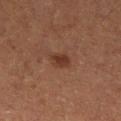diameter=≈2.5 mm | acquisition=15 mm crop, total-body photography | lighting=cross-polarized illumination | subject=female, approximately 50 years of age | anatomic site=the left lower leg | image-analysis metrics=a lesion color around L≈28 a*≈18 b*≈23 in CIELAB and about 7 CIELAB-L* units darker than the surrounding skin; a nevus-likeness score of about 85/100 and lesion-presence confidence of about 100/100.